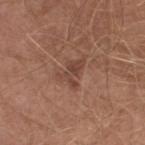| field | value |
|---|---|
| follow-up | imaged on a skin check; not biopsied |
| anatomic site | the right lower leg |
| lesion diameter | about 3.5 mm |
| image-analysis metrics | a classifier nevus-likeness of about 0/100 and a detector confidence of about 100 out of 100 that the crop contains a lesion |
| patient | male, aged 63–67 |
| imaging modality | ~15 mm crop, total-body skin-cancer survey |